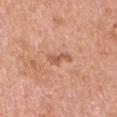The lesion was photographed on a routine skin check and not biopsied; there is no pathology result.
Cropped from a total-body skin-imaging series; the visible field is about 15 mm.
The tile uses white-light illumination.
A male patient, roughly 70 years of age.
Located on the right upper arm.